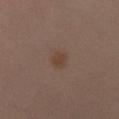notes: total-body-photography surveillance lesion; no biopsy | acquisition: total-body-photography crop, ~15 mm field of view | illumination: cross-polarized | patient: female, aged around 40 | size: about 2.5 mm | site: the left leg.